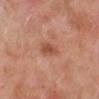Cropped from a total-body skin-imaging series; the visible field is about 15 mm.
About 2.5 mm across.
A male subject aged 78–82.
On the left lower leg.
The tile uses white-light illumination.